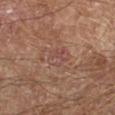The lesion was tiled from a total-body skin photograph and was not biopsied.
The patient is a male aged 63 to 67.
A 15 mm crop from a total-body photograph taken for skin-cancer surveillance.
On the left lower leg.
Captured under cross-polarized illumination.